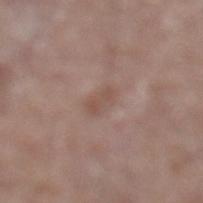Case summary:
- follow-up — no biopsy performed (imaged during a skin exam)
- automated metrics — a border-irregularity rating of about 2.5/10, a color-variation rating of about 3/10, and a peripheral color-asymmetry measure near 1
- image source — ~15 mm crop, total-body skin-cancer survey
- subject — male, approximately 65 years of age
- size — ≈3 mm
- body site — the left lower leg
- illumination — white-light illumination